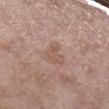Impression: This lesion was catalogued during total-body skin photography and was not selected for biopsy. Acquisition and patient details: A close-up tile cropped from a whole-body skin photograph, about 15 mm across. The lesion is on the left lower leg. About 3.5 mm across. A female patient, roughly 85 years of age or older. Automated tile analysis of the lesion measured an average lesion color of about L≈55 a*≈18 b*≈26 (CIELAB) and about 7 CIELAB-L* units darker than the surrounding skin.